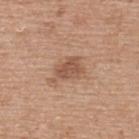notes — catalogued during a skin exam; not biopsied
image — ~15 mm crop, total-body skin-cancer survey
location — the upper back
subject — female, about 60 years old